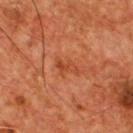Clinical impression: Captured during whole-body skin photography for melanoma surveillance; the lesion was not biopsied. Context: Automated image analysis of the tile measured a lesion area of about 3 mm², an outline eccentricity of about 0.9 (0 = round, 1 = elongated), and a shape-asymmetry score of about 0.5 (0 = symmetric). The analysis additionally found an average lesion color of about L≈43 a*≈30 b*≈37 (CIELAB). It also reported a classifier nevus-likeness of about 0/100 and a lesion-detection confidence of about 100/100. The lesion is on the chest. The subject is a male aged approximately 55. The tile uses cross-polarized illumination. Approximately 3 mm at its widest. Cropped from a whole-body photographic skin survey; the tile spans about 15 mm.